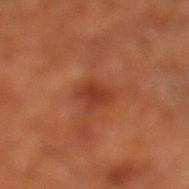Clinical impression: The lesion was tiled from a total-body skin photograph and was not biopsied. Clinical summary: A subject aged 63–67. A lesion tile, about 15 mm wide, cut from a 3D total-body photograph. The lesion is located on the left lower leg. The tile uses cross-polarized illumination.